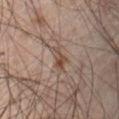Q: Was this lesion biopsied?
A: catalogued during a skin exam; not biopsied
Q: Patient demographics?
A: male, aged 63 to 67
Q: Where on the body is the lesion?
A: the abdomen
Q: What kind of image is this?
A: ~15 mm tile from a whole-body skin photo